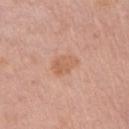Imaged during a routine full-body skin examination; the lesion was not biopsied and no histopathology is available.
A 15 mm close-up tile from a total-body photography series done for melanoma screening.
A female patient about 65 years old.
The lesion is located on the chest.